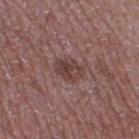  lighting: white-light
  automated_metrics:
    cielab_L: 40
    cielab_a: 20
    cielab_b: 20
    vs_skin_contrast_norm: 7.0
    nevus_likeness_0_100: 25
    lesion_detection_confidence_0_100: 100
  patient:
    sex: male
    age_approx: 50
  lesion_size:
    long_diameter_mm_approx: 3.5
  image:
    source: total-body photography crop
    field_of_view_mm: 15
  site: right thigh